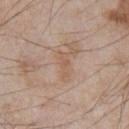{"lesion_size": {"long_diameter_mm_approx": 3.0}, "image": {"source": "total-body photography crop", "field_of_view_mm": 15}, "site": "chest", "patient": {"sex": "male", "age_approx": 65}}